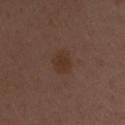Clinical impression:
Recorded during total-body skin imaging; not selected for excision or biopsy.
Background:
From the right upper arm. A female subject aged approximately 50. The tile uses white-light illumination. Measured at roughly 3 mm in maximum diameter. A 15 mm close-up tile from a total-body photography series done for melanoma screening.